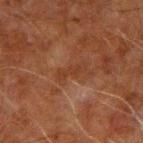Part of a total-body skin-imaging series; this lesion was reviewed on a skin check and was not flagged for biopsy.
A male patient aged 58–62.
Captured under cross-polarized illumination.
From the left arm.
An algorithmic analysis of the crop reported an area of roughly 3 mm², a shape eccentricity near 0.95, and a symmetry-axis asymmetry near 0.4. It also reported an average lesion color of about L≈28 a*≈19 b*≈26 (CIELAB), a lesion–skin lightness drop of about 5, and a lesion-to-skin contrast of about 5.5 (normalized; higher = more distinct). It also reported border irregularity of about 5.5 on a 0–10 scale, internal color variation of about 0 on a 0–10 scale, and a peripheral color-asymmetry measure near 0.
A roughly 15 mm field-of-view crop from a total-body skin photograph.